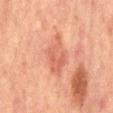Q: Was a biopsy performed?
A: catalogued during a skin exam; not biopsied
Q: What is the anatomic site?
A: the front of the torso
Q: What kind of image is this?
A: ~15 mm crop, total-body skin-cancer survey
Q: Patient demographics?
A: male, aged approximately 65
Q: How was the tile lit?
A: cross-polarized
Q: Lesion size?
A: ~6 mm (longest diameter)